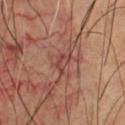| feature | finding |
|---|---|
| tile lighting | cross-polarized illumination |
| patient | male, in their 60s |
| acquisition | total-body-photography crop, ~15 mm field of view |
| location | the chest |